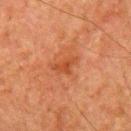Part of a total-body skin-imaging series; this lesion was reviewed on a skin check and was not flagged for biopsy.
Automated image analysis of the tile measured border irregularity of about 4 on a 0–10 scale, a within-lesion color-variation index near 2/10, and a peripheral color-asymmetry measure near 0.5. The software also gave an automated nevus-likeness rating near 0 out of 100 and lesion-presence confidence of about 100/100.
Cropped from a whole-body photographic skin survey; the tile spans about 15 mm.
A male subject roughly 80 years of age.
This is a cross-polarized tile.
Approximately 3.5 mm at its widest.
Located on the left upper arm.